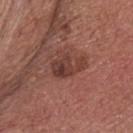workup: total-body-photography surveillance lesion; no biopsy | location: the head or neck | imaging modality: ~15 mm crop, total-body skin-cancer survey | lighting: white-light illumination | image-analysis metrics: a footprint of about 7 mm² and an eccentricity of roughly 0.75; a mean CIELAB color near L≈39 a*≈23 b*≈24 | patient: male, in their mid- to late 70s.